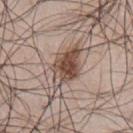{"biopsy_status": "not biopsied; imaged during a skin examination", "site": "chest", "lesion_size": {"long_diameter_mm_approx": 5.5}, "image": {"source": "total-body photography crop", "field_of_view_mm": 15}, "lighting": "white-light", "patient": {"sex": "male", "age_approx": 45}}